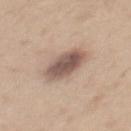  biopsy_status: not biopsied; imaged during a skin examination
  image:
    source: total-body photography crop
    field_of_view_mm: 15
  patient:
    sex: male
    age_approx: 35
  automated_metrics:
    eccentricity: 0.85
    shape_asymmetry: 0.2
  site: mid back